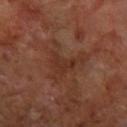{
  "biopsy_status": "not biopsied; imaged during a skin examination",
  "site": "right forearm",
  "lighting": "cross-polarized",
  "image": {
    "source": "total-body photography crop",
    "field_of_view_mm": 15
  },
  "lesion_size": {
    "long_diameter_mm_approx": 3.0
  },
  "patient": {
    "sex": "female",
    "age_approx": 65
  }
}